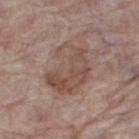Imaged during a routine full-body skin examination; the lesion was not biopsied and no histopathology is available.
Imaged with white-light lighting.
Automated tile analysis of the lesion measured a lesion–skin lightness drop of about 8 and a normalized border contrast of about 6.5. It also reported border irregularity of about 3.5 on a 0–10 scale and a within-lesion color-variation index near 5.5/10.
On the right thigh.
A female subject, aged approximately 85 or older.
A region of skin cropped from a whole-body photographic capture, roughly 15 mm wide.
Longest diameter approximately 6 mm.